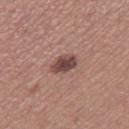This lesion was catalogued during total-body skin photography and was not selected for biopsy.
Cropped from a whole-body photographic skin survey; the tile spans about 15 mm.
A female subject, aged approximately 35.
The lesion is located on the right thigh.